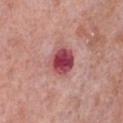The tile uses white-light illumination.
The lesion is located on the chest.
The subject is a female aged 63 to 67.
A lesion tile, about 15 mm wide, cut from a 3D total-body photograph.